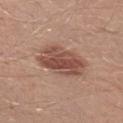Recorded during total-body skin imaging; not selected for excision or biopsy. A male subject, roughly 30 years of age. This image is a 15 mm lesion crop taken from a total-body photograph. The tile uses white-light illumination. On the right lower leg. About 6 mm across.